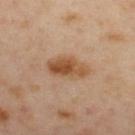follow-up: catalogued during a skin exam; not biopsied
automated lesion analysis: a lesion color around L≈52 a*≈21 b*≈36 in CIELAB, roughly 12 lightness units darker than nearby skin, and a lesion-to-skin contrast of about 9.5 (normalized; higher = more distinct); a border-irregularity rating of about 2.5/10, internal color variation of about 6 on a 0–10 scale, and radial color variation of about 2
imaging modality: ~15 mm tile from a whole-body skin photo
size: ~5 mm (longest diameter)
tile lighting: cross-polarized illumination
patient: female, in their 40s
body site: the upper back